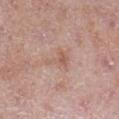Recorded during total-body skin imaging; not selected for excision or biopsy.
Automated image analysis of the tile measured an average lesion color of about L≈58 a*≈20 b*≈27 (CIELAB), about 7 CIELAB-L* units darker than the surrounding skin, and a lesion-to-skin contrast of about 5.5 (normalized; higher = more distinct). The analysis additionally found a lesion-detection confidence of about 100/100.
This is a white-light tile.
A male patient, about 50 years old.
A 15 mm close-up extracted from a 3D total-body photography capture.
On the right lower leg.